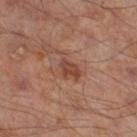Assessment: This lesion was catalogued during total-body skin photography and was not selected for biopsy. Acquisition and patient details: A male subject, aged approximately 65. Cropped from a total-body skin-imaging series; the visible field is about 15 mm. From the leg. The tile uses cross-polarized illumination. Approximately 3.5 mm at its widest. The lesion-visualizer software estimated an area of roughly 6 mm² and a shape-asymmetry score of about 0.4 (0 = symmetric). The software also gave a classifier nevus-likeness of about 15/100 and lesion-presence confidence of about 100/100.